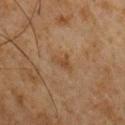<lesion>
<biopsy_status>not biopsied; imaged during a skin examination</biopsy_status>
<image>
  <source>total-body photography crop</source>
  <field_of_view_mm>15</field_of_view_mm>
</image>
<patient>
  <sex>male</sex>
  <age_approx>60</age_approx>
</patient>
<lesion_size>
  <long_diameter_mm_approx>3.0</long_diameter_mm_approx>
</lesion_size>
<site>chest</site>
</lesion>